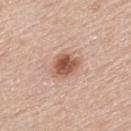| field | value |
|---|---|
| workup | catalogued during a skin exam; not biopsied |
| diameter | about 4 mm |
| lighting | white-light illumination |
| subject | male, aged approximately 60 |
| image | ~15 mm tile from a whole-body skin photo |
| site | the upper back |